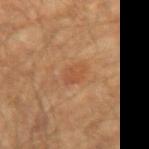follow-up: total-body-photography surveillance lesion; no biopsy
diameter: ≈3 mm
automated metrics: a lesion area of about 5 mm² and a shape eccentricity near 0.75
patient: male, aged 63–67
location: the left upper arm
acquisition: total-body-photography crop, ~15 mm field of view